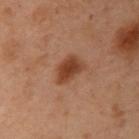- notes · total-body-photography surveillance lesion; no biopsy
- image source · total-body-photography crop, ~15 mm field of view
- size · ~3.5 mm (longest diameter)
- patient · female, roughly 55 years of age
- anatomic site · the arm